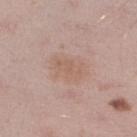Impression:
Recorded during total-body skin imaging; not selected for excision or biopsy.
Image and clinical context:
Located on the right lower leg. A male patient in their 40s. Imaged with white-light lighting. A 15 mm crop from a total-body photograph taken for skin-cancer surveillance. Longest diameter approximately 4.5 mm.